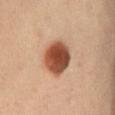workup — imaged on a skin check; not biopsied
acquisition — 15 mm crop, total-body photography
patient — female, aged around 35
site — the chest
lesion size — ≈4.5 mm